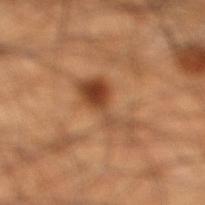Assessment:
Captured during whole-body skin photography for melanoma surveillance; the lesion was not biopsied.
Context:
Located on the right lower leg. A lesion tile, about 15 mm wide, cut from a 3D total-body photograph. The tile uses cross-polarized illumination. The total-body-photography lesion software estimated a shape-asymmetry score of about 0.65 (0 = symmetric). The software also gave a normalized lesion–skin contrast near 8.5. The software also gave a detector confidence of about 100 out of 100 that the crop contains a lesion. A male subject, about 45 years old.